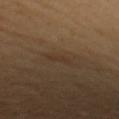A 15 mm crop from a total-body photograph taken for skin-cancer surveillance. The lesion is on the arm. A female patient, aged around 55. Measured at roughly 2.5 mm in maximum diameter. This is a cross-polarized tile. An algorithmic analysis of the crop reported a mean CIELAB color near L≈34 a*≈14 b*≈27, roughly 4 lightness units darker than nearby skin, and a normalized lesion–skin contrast near 4.5.